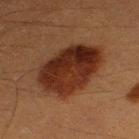Impression:
Recorded during total-body skin imaging; not selected for excision or biopsy.
Image and clinical context:
A roughly 15 mm field-of-view crop from a total-body skin photograph. On the left thigh. A male subject, approximately 40 years of age.